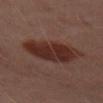– workup · total-body-photography surveillance lesion; no biopsy
– lighting · cross-polarized
– patient · female, aged approximately 50
– image · 15 mm crop, total-body photography
– anatomic site · the leg
– automated lesion analysis · an average lesion color of about L≈28 a*≈20 b*≈22 (CIELAB) and a lesion–skin lightness drop of about 10; internal color variation of about 3.5 on a 0–10 scale and radial color variation of about 1; a nevus-likeness score of about 100/100 and lesion-presence confidence of about 100/100
– size · about 6.5 mm